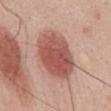Impression: Part of a total-body skin-imaging series; this lesion was reviewed on a skin check and was not flagged for biopsy. Acquisition and patient details: An algorithmic analysis of the crop reported a footprint of about 25 mm², an outline eccentricity of about 0.7 (0 = round, 1 = elongated), and a symmetry-axis asymmetry near 0.1. The analysis additionally found a mean CIELAB color near L≈54 a*≈26 b*≈27, roughly 14 lightness units darker than nearby skin, and a normalized border contrast of about 9. And it measured a within-lesion color-variation index near 4/10. Captured under white-light illumination. The recorded lesion diameter is about 6.5 mm. A 15 mm close-up tile from a total-body photography series done for melanoma screening. Located on the mid back. A male patient, aged 53 to 57.